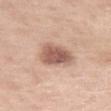Q: Is there a histopathology result?
A: imaged on a skin check; not biopsied
Q: How was this image acquired?
A: total-body-photography crop, ~15 mm field of view
Q: Automated lesion metrics?
A: an area of roughly 10 mm², an outline eccentricity of about 0.7 (0 = round, 1 = elongated), and a shape-asymmetry score of about 0.25 (0 = symmetric); border irregularity of about 2.5 on a 0–10 scale, internal color variation of about 4 on a 0–10 scale, and a peripheral color-asymmetry measure near 1.5; an automated nevus-likeness rating near 50 out of 100 and a lesion-detection confidence of about 100/100
Q: What are the patient's age and sex?
A: female, in their mid-50s
Q: Where on the body is the lesion?
A: the left thigh